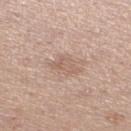Part of a total-body skin-imaging series; this lesion was reviewed on a skin check and was not flagged for biopsy.
The lesion's longest dimension is about 3.5 mm.
The subject is a female aged 28 to 32.
A roughly 15 mm field-of-view crop from a total-body skin photograph.
Imaged with white-light lighting.
Located on the leg.